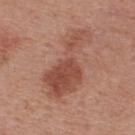biopsy_status: not biopsied; imaged during a skin examination
lighting: white-light
image:
  source: total-body photography crop
  field_of_view_mm: 15
site: upper back
patient:
  sex: male
  age_approx: 55
lesion_size:
  long_diameter_mm_approx: 8.5